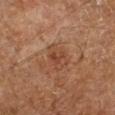<tbp_lesion>
<biopsy_status>not biopsied; imaged during a skin examination</biopsy_status>
<lesion_size>
  <long_diameter_mm_approx>3.5</long_diameter_mm_approx>
</lesion_size>
<patient>
  <age_approx>65</age_approx>
</patient>
<site>left lower leg</site>
<lighting>cross-polarized</lighting>
<image>
  <source>total-body photography crop</source>
  <field_of_view_mm>15</field_of_view_mm>
</image>
</tbp_lesion>